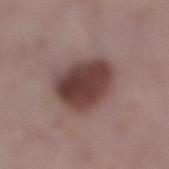follow-up — imaged on a skin check; not biopsied
patient — male, roughly 40 years of age
imaging modality — total-body-photography crop, ~15 mm field of view
automated lesion analysis — a lesion area of about 19 mm² and an outline eccentricity of about 0.8 (0 = round, 1 = elongated); about 16 CIELAB-L* units darker than the surrounding skin and a normalized border contrast of about 12; a classifier nevus-likeness of about 95/100 and a lesion-detection confidence of about 100/100
size — ≈6.5 mm
location — the right lower leg